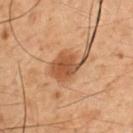| field | value |
|---|---|
| body site | the arm |
| lighting | cross-polarized illumination |
| automated metrics | a border-irregularity rating of about 2.5/10, internal color variation of about 3.5 on a 0–10 scale, and radial color variation of about 1; a classifier nevus-likeness of about 95/100 |
| diameter | ≈5 mm |
| image | 15 mm crop, total-body photography |
| subject | male, aged around 50 |